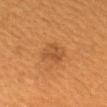Impression: No biopsy was performed on this lesion — it was imaged during a full skin examination and was not determined to be concerning.